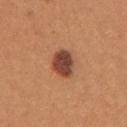biopsy_status: not biopsied; imaged during a skin examination
lighting: white-light
site: right upper arm
automated_metrics:
  cielab_L: 45
  cielab_a: 25
  cielab_b: 31
  vs_skin_darker_L: 15.0
  vs_skin_contrast_norm: 11.5
image:
  source: total-body photography crop
  field_of_view_mm: 15
patient:
  sex: female
  age_approx: 25
lesion_size:
  long_diameter_mm_approx: 3.5